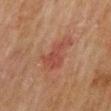This image is a 15 mm lesion crop taken from a total-body photograph. The tile uses cross-polarized illumination. An algorithmic analysis of the crop reported a lesion color around L≈38 a*≈23 b*≈25 in CIELAB, roughly 6 lightness units darker than nearby skin, and a normalized border contrast of about 5.5. The analysis additionally found an automated nevus-likeness rating near 50 out of 100 and lesion-presence confidence of about 100/100. The lesion's longest dimension is about 4.5 mm. A male patient, aged approximately 70. The lesion is on the mid back.